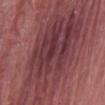Clinical impression:
Captured during whole-body skin photography for melanoma surveillance; the lesion was not biopsied.
Context:
On the abdomen. A 15 mm close-up extracted from a 3D total-body photography capture. About 19 mm across. Imaged with white-light lighting. A male subject, aged approximately 40.